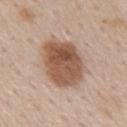The lesion was tiled from a total-body skin photograph and was not biopsied. Measured at roughly 6.5 mm in maximum diameter. The lesion is located on the back. Captured under white-light illumination. Cropped from a whole-body photographic skin survey; the tile spans about 15 mm. A male subject in their 60s. The lesion-visualizer software estimated a nevus-likeness score of about 100/100 and a detector confidence of about 100 out of 100 that the crop contains a lesion.